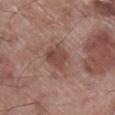The lesion was photographed on a routine skin check and not biopsied; there is no pathology result. The subject is a male about 70 years old. This is a white-light tile. Located on the leg. Approximately 4 mm at its widest. A region of skin cropped from a whole-body photographic capture, roughly 15 mm wide.